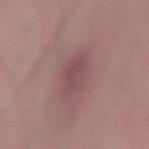Case summary:
- notes — catalogued during a skin exam; not biopsied
- patient — male, in their mid- to late 60s
- automated lesion analysis — a within-lesion color-variation index near 2/10 and a peripheral color-asymmetry measure near 0.5; a classifier nevus-likeness of about 0/100 and a detector confidence of about 90 out of 100 that the crop contains a lesion
- location — the lower back
- acquisition — total-body-photography crop, ~15 mm field of view
- size — ≈3.5 mm